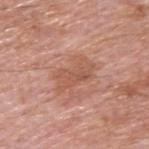Assessment: Imaged during a routine full-body skin examination; the lesion was not biopsied and no histopathology is available. Image and clinical context: A male patient, roughly 60 years of age. This image is a 15 mm lesion crop taken from a total-body photograph. On the upper back. Automated tile analysis of the lesion measured an area of roughly 10 mm² and a shape eccentricity near 0.8. And it measured a mean CIELAB color near L≈55 a*≈24 b*≈30 and a normalized border contrast of about 5.5. It also reported border irregularity of about 4.5 on a 0–10 scale and a peripheral color-asymmetry measure near 1. And it measured a detector confidence of about 100 out of 100 that the crop contains a lesion.